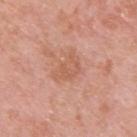Acquisition and patient details:
This image is a 15 mm lesion crop taken from a total-body photograph. Imaged with white-light lighting. A male subject, aged approximately 40. Located on the upper back. The lesion's longest dimension is about 3.5 mm. Automated image analysis of the tile measured an area of roughly 7.5 mm² and a symmetry-axis asymmetry near 0.45. The software also gave internal color variation of about 2.5 on a 0–10 scale and a peripheral color-asymmetry measure near 0.5. The software also gave a nevus-likeness score of about 0/100 and a lesion-detection confidence of about 100/100.